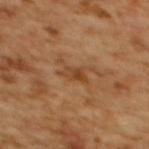* biopsy status — no biopsy performed (imaged during a skin exam)
* lesion size — ≈3.5 mm
* tile lighting — cross-polarized
* patient — female, approximately 55 years of age
* site — the back
* image-analysis metrics — a normalized border contrast of about 6.5; a border-irregularity rating of about 5/10 and internal color variation of about 4.5 on a 0–10 scale; an automated nevus-likeness rating near 0 out of 100 and a detector confidence of about 100 out of 100 that the crop contains a lesion
* acquisition — 15 mm crop, total-body photography